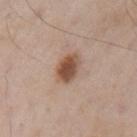follow-up = imaged on a skin check; not biopsied | diameter = ~3.5 mm (longest diameter) | illumination = white-light illumination | patient = male, approximately 55 years of age | image source = ~15 mm crop, total-body skin-cancer survey | site = the left upper arm.